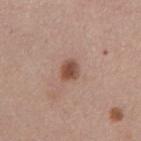Captured during whole-body skin photography for melanoma surveillance; the lesion was not biopsied. The total-body-photography lesion software estimated a lesion area of about 4.5 mm², an eccentricity of roughly 0.6, and two-axis asymmetry of about 0.25. It also reported a border-irregularity index near 2/10 and a peripheral color-asymmetry measure near 1. It also reported a detector confidence of about 100 out of 100 that the crop contains a lesion. This is a white-light tile. A 15 mm close-up extracted from a 3D total-body photography capture. The lesion's longest dimension is about 2.5 mm. A male patient, about 55 years old. Located on the abdomen.